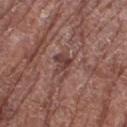Assessment:
This lesion was catalogued during total-body skin photography and was not selected for biopsy.
Background:
Automated image analysis of the tile measured a border-irregularity rating of about 5.5/10, a within-lesion color-variation index near 1.5/10, and peripheral color asymmetry of about 0.5. The software also gave a detector confidence of about 60 out of 100 that the crop contains a lesion. A female patient, roughly 80 years of age. A lesion tile, about 15 mm wide, cut from a 3D total-body photograph. The lesion's longest dimension is about 3 mm. From the left thigh. The tile uses white-light illumination.